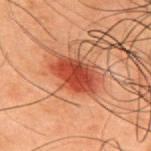follow-up: total-body-photography surveillance lesion; no biopsy | patient: male, aged approximately 50 | location: the upper back | acquisition: ~15 mm crop, total-body skin-cancer survey | tile lighting: cross-polarized illumination | lesion diameter: ~5 mm (longest diameter).